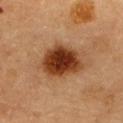About 5.5 mm across. Captured under cross-polarized illumination. A female patient, about 60 years old. A 15 mm close-up tile from a total-body photography series done for melanoma screening. The lesion is located on the upper back.